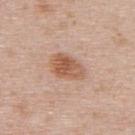Findings:
* biopsy status: imaged on a skin check; not biopsied
* anatomic site: the upper back
* patient: male, in their 40s
* imaging modality: 15 mm crop, total-body photography